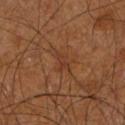The lesion was tiled from a total-body skin photograph and was not biopsied. A male patient aged approximately 65. Imaged with cross-polarized lighting. A 15 mm close-up tile from a total-body photography series done for melanoma screening. About 3 mm across. The lesion is located on the right upper arm.